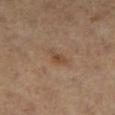Impression: The lesion was photographed on a routine skin check and not biopsied; there is no pathology result. Image and clinical context: The patient is a female roughly 40 years of age. From the leg. A region of skin cropped from a whole-body photographic capture, roughly 15 mm wide.